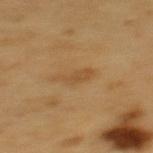Clinical impression:
The lesion was photographed on a routine skin check and not biopsied; there is no pathology result.
Image and clinical context:
A male patient in their 60s. A lesion tile, about 15 mm wide, cut from a 3D total-body photograph. Located on the mid back.